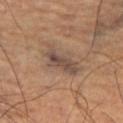follow-up — no biopsy performed (imaged during a skin exam)
patient — male, about 70 years old
imaging modality — total-body-photography crop, ~15 mm field of view
lesion size — ~5 mm (longest diameter)
body site — the right thigh
TBP lesion metrics — a mean CIELAB color near L≈45 a*≈15 b*≈24, a lesion–skin lightness drop of about 8, and a normalized lesion–skin contrast near 7.5; a border-irregularity rating of about 4.5/10, a color-variation rating of about 4.5/10, and radial color variation of about 1.5
lighting — cross-polarized